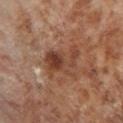Findings:
- notes · no biopsy performed (imaged during a skin exam)
- tile lighting · cross-polarized
- image source · 15 mm crop, total-body photography
- subject · female, aged 53–57
- location · the leg